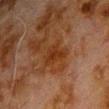The lesion was photographed on a routine skin check and not biopsied; there is no pathology result.
Longest diameter approximately 3 mm.
A male subject in their 80s.
The tile uses cross-polarized illumination.
The lesion is located on the chest.
This image is a 15 mm lesion crop taken from a total-body photograph.